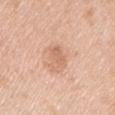The patient is a male about 45 years old.
The lesion is on the left upper arm.
A roughly 15 mm field-of-view crop from a total-body skin photograph.
The tile uses white-light illumination.